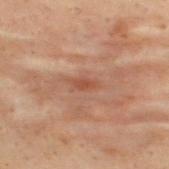Imaged with cross-polarized lighting. An algorithmic analysis of the crop reported a footprint of about 2.5 mm² and a symmetry-axis asymmetry near 0.25. And it measured a lesion color around L≈40 a*≈21 b*≈27 in CIELAB, about 7 CIELAB-L* units darker than the surrounding skin, and a normalized border contrast of about 6. The software also gave a border-irregularity rating of about 2.5/10, internal color variation of about 0.5 on a 0–10 scale, and a peripheral color-asymmetry measure near 0. A region of skin cropped from a whole-body photographic capture, roughly 15 mm wide. The lesion is on the upper back. The subject is a male aged approximately 30. About 2.5 mm across.